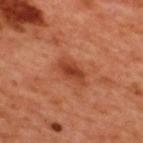Assessment: The lesion was tiled from a total-body skin photograph and was not biopsied. Context: A male subject, aged around 50. Imaged with cross-polarized lighting. A 15 mm close-up extracted from a 3D total-body photography capture. The lesion is located on the upper back.